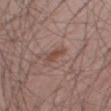Assessment: Part of a total-body skin-imaging series; this lesion was reviewed on a skin check and was not flagged for biopsy. Context: This is a white-light tile. On the leg. Approximately 2.5 mm at its widest. A 15 mm close-up extracted from a 3D total-body photography capture. The subject is a male approximately 55 years of age. The lesion-visualizer software estimated a border-irregularity index near 3/10 and a color-variation rating of about 0.5/10.